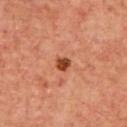Recorded during total-body skin imaging; not selected for excision or biopsy.
A 15 mm close-up extracted from a 3D total-body photography capture.
The lesion's longest dimension is about 2 mm.
From the upper back.
The subject is a male roughly 70 years of age.
The tile uses cross-polarized illumination.